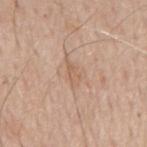This lesion was catalogued during total-body skin photography and was not selected for biopsy.
Approximately 3.5 mm at its widest.
Imaged with white-light lighting.
The subject is a male aged 63–67.
Cropped from a whole-body photographic skin survey; the tile spans about 15 mm.
Located on the mid back.